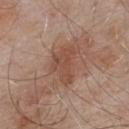The lesion was tiled from a total-body skin photograph and was not biopsied.
On the upper back.
A male patient aged around 55.
The recorded lesion diameter is about 4.5 mm.
Captured under white-light illumination.
A close-up tile cropped from a whole-body skin photograph, about 15 mm across.